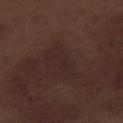• follow-up: no biopsy performed (imaged during a skin exam)
• lesion diameter: about 5 mm
• tile lighting: white-light illumination
• patient: male, aged around 70
• automated metrics: a lesion area of about 6.5 mm² and two-axis asymmetry of about 0.45
• image source: ~15 mm crop, total-body skin-cancer survey
• location: the right lower leg